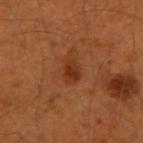Case summary:
* notes — total-body-photography surveillance lesion; no biopsy
* image source — ~15 mm crop, total-body skin-cancer survey
* tile lighting — cross-polarized
* size — about 2.5 mm
* anatomic site — the left arm
* patient — male, about 50 years old